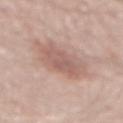This lesion was catalogued during total-body skin photography and was not selected for biopsy. A male patient, about 70 years old. This image is a 15 mm lesion crop taken from a total-body photograph. Automated tile analysis of the lesion measured a footprint of about 15 mm², a shape eccentricity near 0.75, and two-axis asymmetry of about 0.2. And it measured border irregularity of about 2.5 on a 0–10 scale, a color-variation rating of about 3.5/10, and peripheral color asymmetry of about 1. And it measured a classifier nevus-likeness of about 35/100 and a detector confidence of about 100 out of 100 that the crop contains a lesion. The lesion is located on the mid back. About 5.5 mm across. Captured under white-light illumination.